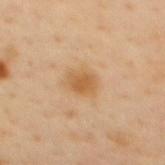Impression:
Captured during whole-body skin photography for melanoma surveillance; the lesion was not biopsied.
Context:
A 15 mm crop from a total-body photograph taken for skin-cancer surveillance. From the mid back. A male subject, about 40 years old.